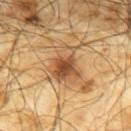biopsy status = catalogued during a skin exam; not biopsied | automated lesion analysis = a footprint of about 13 mm² and a symmetry-axis asymmetry near 0.35; a nevus-likeness score of about 75/100 and a lesion-detection confidence of about 100/100 | lesion size = ≈4.5 mm | imaging modality = 15 mm crop, total-body photography | location = the upper back | patient = male, aged approximately 65.